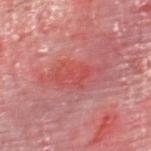Q: Was a biopsy performed?
A: imaged on a skin check; not biopsied
Q: Patient demographics?
A: male, aged approximately 55
Q: What is the imaging modality?
A: ~15 mm tile from a whole-body skin photo
Q: Illumination type?
A: cross-polarized
Q: What is the anatomic site?
A: the right thigh
Q: Lesion size?
A: about 5.5 mm